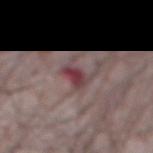The lesion was tiled from a total-body skin photograph and was not biopsied. A close-up tile cropped from a whole-body skin photograph, about 15 mm across. The tile uses white-light illumination. The lesion's longest dimension is about 3 mm. Located on the abdomen. A male patient roughly 75 years of age.